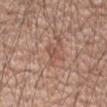{"image": {"source": "total-body photography crop", "field_of_view_mm": 15}, "site": "left upper arm", "automated_metrics": {"area_mm2_approx": 3.0, "eccentricity": 0.8, "shape_asymmetry": 0.45, "vs_skin_darker_L": 7.0, "vs_skin_contrast_norm": 5.0, "border_irregularity_0_10": 4.0, "peripheral_color_asymmetry": 0.5}, "patient": {"sex": "male", "age_approx": 50}, "lighting": "white-light"}